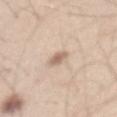notes = catalogued during a skin exam; not biopsied | diameter = about 3 mm | illumination = white-light illumination | image source = ~15 mm crop, total-body skin-cancer survey | image-analysis metrics = a footprint of about 3.5 mm², a shape eccentricity near 0.85, and two-axis asymmetry of about 0.35; a lesion color around L≈64 a*≈16 b*≈27 in CIELAB, roughly 12 lightness units darker than nearby skin, and a normalized lesion–skin contrast near 7.5 | anatomic site = the mid back | subject = male, approximately 60 years of age.